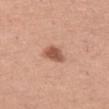Imaged during a routine full-body skin examination; the lesion was not biopsied and no histopathology is available.
The lesion's longest dimension is about 2.5 mm.
A lesion tile, about 15 mm wide, cut from a 3D total-body photograph.
Captured under white-light illumination.
The subject is a female aged around 50.
The lesion is on the left thigh.